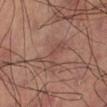{"lesion_size": {"long_diameter_mm_approx": 4.0}, "image": {"source": "total-body photography crop", "field_of_view_mm": 15}, "patient": {"sex": "male", "age_approx": 60}}